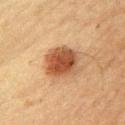Assessment:
Captured during whole-body skin photography for melanoma surveillance; the lesion was not biopsied.
Image and clinical context:
From the chest. A male patient, roughly 60 years of age. The recorded lesion diameter is about 4 mm. A roughly 15 mm field-of-view crop from a total-body skin photograph.